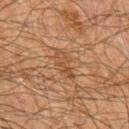Impression: This lesion was catalogued during total-body skin photography and was not selected for biopsy. Clinical summary: Imaged with cross-polarized lighting. The lesion is located on the chest. Automated image analysis of the tile measured a lesion color around L≈38 a*≈17 b*≈28 in CIELAB and a normalized border contrast of about 5. The analysis additionally found a detector confidence of about 65 out of 100 that the crop contains a lesion. A male patient approximately 60 years of age. A 15 mm close-up extracted from a 3D total-body photography capture. The lesion's longest dimension is about 4 mm.